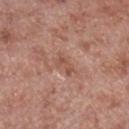Assessment: Captured during whole-body skin photography for melanoma surveillance; the lesion was not biopsied. Context: The recorded lesion diameter is about 2.5 mm. Cropped from a whole-body photographic skin survey; the tile spans about 15 mm. A male subject, aged around 55. The lesion-visualizer software estimated a border-irregularity index near 3.5/10, a within-lesion color-variation index near 2/10, and radial color variation of about 0.5. The analysis additionally found a lesion-detection confidence of about 100/100. Located on the leg. Imaged with white-light lighting.